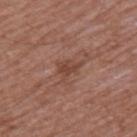Impression: Recorded during total-body skin imaging; not selected for excision or biopsy. Background: An algorithmic analysis of the crop reported a lesion color around L≈44 a*≈21 b*≈27 in CIELAB, a lesion–skin lightness drop of about 8, and a normalized lesion–skin contrast near 6.5. Captured under white-light illumination. A male patient, approximately 55 years of age. Measured at roughly 3 mm in maximum diameter. A lesion tile, about 15 mm wide, cut from a 3D total-body photograph. From the left upper arm.